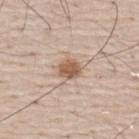follow-up = catalogued during a skin exam; not biopsied | size = ≈2.5 mm | site = the back | image source = ~15 mm crop, total-body skin-cancer survey | tile lighting = white-light | subject = male, aged around 75.